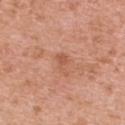<case>
<biopsy_status>not biopsied; imaged during a skin examination</biopsy_status>
<lighting>white-light</lighting>
<image>
  <source>total-body photography crop</source>
  <field_of_view_mm>15</field_of_view_mm>
</image>
<site>left upper arm</site>
<patient>
  <sex>male</sex>
  <age_approx>45</age_approx>
</patient>
<automated_metrics>
  <area_mm2_approx>3.0</area_mm2_approx>
  <shape_asymmetry>0.5</shape_asymmetry>
  <cielab_L>56</cielab_L>
  <cielab_a>26</cielab_a>
  <cielab_b>33</cielab_b>
  <vs_skin_darker_L>8.0</vs_skin_darker_L>
  <border_irregularity_0_10>5.0</border_irregularity_0_10>
  <color_variation_0_10>0.5</color_variation_0_10>
  <peripheral_color_asymmetry>0.0</peripheral_color_asymmetry>
  <nevus_likeness_0_100>0</nevus_likeness_0_100>
  <lesion_detection_confidence_0_100>100</lesion_detection_confidence_0_100>
</automated_metrics>
<lesion_size>
  <long_diameter_mm_approx>3.0</long_diameter_mm_approx>
</lesion_size>
</case>